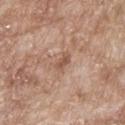Assessment: Captured during whole-body skin photography for melanoma surveillance; the lesion was not biopsied. Context: Automated tile analysis of the lesion measured a footprint of about 3.5 mm² and a symmetry-axis asymmetry near 0.25. The software also gave a lesion color around L≈54 a*≈20 b*≈29 in CIELAB and about 9 CIELAB-L* units darker than the surrounding skin. And it measured an automated nevus-likeness rating near 0 out of 100 and lesion-presence confidence of about 100/100. The tile uses white-light illumination. The subject is a male in their mid-60s. The lesion is located on the upper back. Approximately 2.5 mm at its widest. Cropped from a whole-body photographic skin survey; the tile spans about 15 mm.